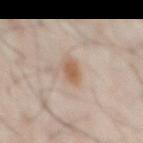Clinical impression: Part of a total-body skin-imaging series; this lesion was reviewed on a skin check and was not flagged for biopsy. Background: A close-up tile cropped from a whole-body skin photograph, about 15 mm across. The lesion is on the chest. The lesion's longest dimension is about 2.5 mm. A male patient aged around 50. The tile uses cross-polarized illumination.